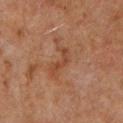biopsy_status: not biopsied; imaged during a skin examination
lighting: cross-polarized
site: upper back
patient:
  sex: male
  age_approx: 60
automated_metrics:
  area_mm2_approx: 3.0
  shape_asymmetry: 0.7
  cielab_L: 40
  cielab_a: 22
  cielab_b: 31
  vs_skin_darker_L: 7.0
  vs_skin_contrast_norm: 6.0
image:
  source: total-body photography crop
  field_of_view_mm: 15